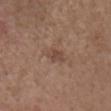Assessment: Part of a total-body skin-imaging series; this lesion was reviewed on a skin check and was not flagged for biopsy. Image and clinical context: A 15 mm crop from a total-body photograph taken for skin-cancer surveillance. The lesion-visualizer software estimated a lesion area of about 4 mm² and a symmetry-axis asymmetry near 0.4. And it measured roughly 7 lightness units darker than nearby skin. The analysis additionally found a border-irregularity rating of about 4/10. And it measured a nevus-likeness score of about 0/100 and a lesion-detection confidence of about 100/100. A female patient, aged 83 or older. Captured under white-light illumination. From the chest.